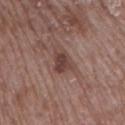workup — no biopsy performed (imaged during a skin exam); size — ~2.5 mm (longest diameter); subject — female, about 70 years old; TBP lesion metrics — a color-variation rating of about 2.5/10 and a peripheral color-asymmetry measure near 1; image source — ~15 mm tile from a whole-body skin photo; illumination — white-light illumination; anatomic site — the right upper arm.